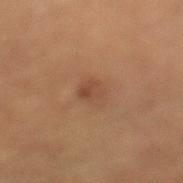patient — female, roughly 80 years of age | automated metrics — about 6 CIELAB-L* units darker than the surrounding skin; a classifier nevus-likeness of about 20/100 and a lesion-detection confidence of about 100/100 | lesion diameter — ≈2.5 mm | site — the leg | tile lighting — cross-polarized | imaging modality — 15 mm crop, total-body photography.